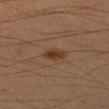Captured during whole-body skin photography for melanoma surveillance; the lesion was not biopsied. The recorded lesion diameter is about 3 mm. A male subject aged 33–37. The lesion is on the left forearm. Imaged with cross-polarized lighting. A lesion tile, about 15 mm wide, cut from a 3D total-body photograph.